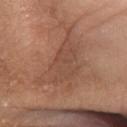{
  "biopsy_status": "not biopsied; imaged during a skin examination",
  "patient": {
    "sex": "female",
    "age_approx": 75
  },
  "site": "left lower leg",
  "automated_metrics": {
    "area_mm2_approx": 23.0,
    "eccentricity": 0.8,
    "shape_asymmetry": 0.55,
    "border_irregularity_0_10": 7.5,
    "color_variation_0_10": 2.5,
    "peripheral_color_asymmetry": 1.0
  },
  "image": {
    "source": "total-body photography crop",
    "field_of_view_mm": 15
  },
  "lighting": "white-light"
}